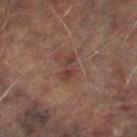Located on the left lower leg. The total-body-photography lesion software estimated an average lesion color of about L≈31 a*≈17 b*≈20 (CIELAB) and a lesion–skin lightness drop of about 6. The analysis additionally found a border-irregularity index near 6.5/10, a within-lesion color-variation index near 0/10, and a peripheral color-asymmetry measure near 0. The software also gave a classifier nevus-likeness of about 0/100 and lesion-presence confidence of about 100/100. The subject is a male approximately 75 years of age. A 15 mm close-up tile from a total-body photography series done for melanoma screening. This is a cross-polarized tile. Measured at roughly 3 mm in maximum diameter.